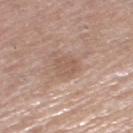Assessment: The lesion was tiled from a total-body skin photograph and was not biopsied. Background: This image is a 15 mm lesion crop taken from a total-body photograph. Automated image analysis of the tile measured a footprint of about 5.5 mm² and two-axis asymmetry of about 0.25. A female subject, about 65 years old. Captured under white-light illumination. Measured at roughly 2.5 mm in maximum diameter. On the left thigh.